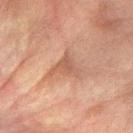Captured during whole-body skin photography for melanoma surveillance; the lesion was not biopsied. A male patient roughly 75 years of age. Automated tile analysis of the lesion measured a shape eccentricity near 0.55 and two-axis asymmetry of about 0.55. It also reported lesion-presence confidence of about 55/100. A lesion tile, about 15 mm wide, cut from a 3D total-body photograph. The recorded lesion diameter is about 3.5 mm. The lesion is on the right forearm.